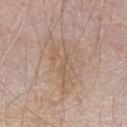subject — male, aged 63–67 | acquisition — total-body-photography crop, ~15 mm field of view | lighting — white-light illumination | location — the left forearm.